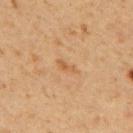biopsy status: imaged on a skin check; not biopsied | location: the upper back | lesion size: ≈2.5 mm | acquisition: total-body-photography crop, ~15 mm field of view | lighting: cross-polarized | patient: male, aged approximately 60.